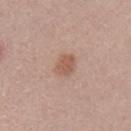Impression:
The lesion was tiled from a total-body skin photograph and was not biopsied.
Context:
The tile uses white-light illumination. The lesion-visualizer software estimated a lesion color around L≈56 a*≈20 b*≈28 in CIELAB and a lesion–skin lightness drop of about 9. It also reported a border-irregularity rating of about 1.5/10, a within-lesion color-variation index near 2/10, and peripheral color asymmetry of about 0.5. The software also gave a classifier nevus-likeness of about 75/100 and a detector confidence of about 100 out of 100 that the crop contains a lesion. A male subject, aged around 30. A region of skin cropped from a whole-body photographic capture, roughly 15 mm wide.